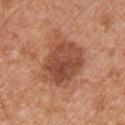A male subject, aged approximately 30.
Imaged with white-light lighting.
The lesion-visualizer software estimated a mean CIELAB color near L≈49 a*≈25 b*≈32, a lesion–skin lightness drop of about 12, and a lesion-to-skin contrast of about 8.5 (normalized; higher = more distinct). The analysis additionally found a border-irregularity index near 3/10, internal color variation of about 5.5 on a 0–10 scale, and radial color variation of about 2.
A lesion tile, about 15 mm wide, cut from a 3D total-body photograph.
The lesion's longest dimension is about 6.5 mm.
The lesion is on the chest.